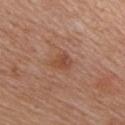follow-up: catalogued during a skin exam; not biopsied
image: total-body-photography crop, ~15 mm field of view
TBP lesion metrics: an area of roughly 4 mm², an outline eccentricity of about 0.6 (0 = round, 1 = elongated), and a shape-asymmetry score of about 0.3 (0 = symmetric); a mean CIELAB color near L≈48 a*≈23 b*≈32, roughly 8 lightness units darker than nearby skin, and a lesion-to-skin contrast of about 6.5 (normalized; higher = more distinct); border irregularity of about 2.5 on a 0–10 scale, a within-lesion color-variation index near 3.5/10, and peripheral color asymmetry of about 1.5
patient: male, aged 58 to 62
diameter: ~2.5 mm (longest diameter)
lighting: white-light illumination
body site: the chest